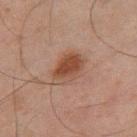Assessment:
The lesion was photographed on a routine skin check and not biopsied; there is no pathology result.
Background:
A male subject approximately 45 years of age. A lesion tile, about 15 mm wide, cut from a 3D total-body photograph. Imaged with cross-polarized lighting. Located on the right thigh. The recorded lesion diameter is about 4.5 mm. An algorithmic analysis of the crop reported an area of roughly 10 mm², an eccentricity of roughly 0.75, and two-axis asymmetry of about 0.15. The software also gave a border-irregularity rating of about 2/10, internal color variation of about 4.5 on a 0–10 scale, and radial color variation of about 1.5.